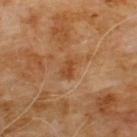<lesion>
  <biopsy_status>not biopsied; imaged during a skin examination</biopsy_status>
  <lesion_size>
    <long_diameter_mm_approx>2.5</long_diameter_mm_approx>
  </lesion_size>
  <site>chest</site>
  <patient>
    <sex>male</sex>
    <age_approx>60</age_approx>
  </patient>
  <lighting>cross-polarized</lighting>
  <image>
    <source>total-body photography crop</source>
    <field_of_view_mm>15</field_of_view_mm>
  </image>
</lesion>